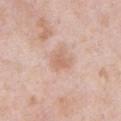Clinical impression:
Part of a total-body skin-imaging series; this lesion was reviewed on a skin check and was not flagged for biopsy.
Context:
On the abdomen. The subject is a male aged 53 to 57. The total-body-photography lesion software estimated an area of roughly 5.5 mm², a shape eccentricity near 0.35, and a symmetry-axis asymmetry near 0.3. And it measured a lesion color around L≈65 a*≈20 b*≈29 in CIELAB, a lesion–skin lightness drop of about 8, and a normalized border contrast of about 5.5. It also reported an automated nevus-likeness rating near 0 out of 100. A close-up tile cropped from a whole-body skin photograph, about 15 mm across. The lesion's longest dimension is about 2.5 mm.